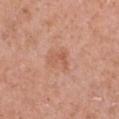workup: imaged on a skin check; not biopsied | anatomic site: the chest | image: total-body-photography crop, ~15 mm field of view | patient: female, roughly 40 years of age.